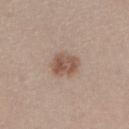Part of a total-body skin-imaging series; this lesion was reviewed on a skin check and was not flagged for biopsy. Automated tile analysis of the lesion measured a lesion area of about 7.5 mm², an outline eccentricity of about 0.6 (0 = round, 1 = elongated), and a shape-asymmetry score of about 0.2 (0 = symmetric). It also reported a border-irregularity index near 1.5/10 and a color-variation rating of about 4/10. It also reported a nevus-likeness score of about 85/100 and lesion-presence confidence of about 100/100. On the right lower leg. A 15 mm close-up extracted from a 3D total-body photography capture. This is a white-light tile. The patient is a female aged 38–42.